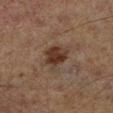workup: no biopsy performed (imaged during a skin exam) | diameter: ≈4 mm | anatomic site: the left lower leg | tile lighting: cross-polarized | imaging modality: 15 mm crop, total-body photography | automated lesion analysis: an eccentricity of roughly 0.7 and a shape-asymmetry score of about 0.3 (0 = symmetric); a mean CIELAB color near L≈31 a*≈16 b*≈23, a lesion–skin lightness drop of about 9, and a normalized lesion–skin contrast near 9; a border-irregularity rating of about 3/10; an automated nevus-likeness rating near 85 out of 100 and lesion-presence confidence of about 100/100 | patient: male, aged 63 to 67.